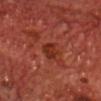Findings:
– imaging modality: ~15 mm tile from a whole-body skin photo
– diameter: ~3 mm (longest diameter)
– tile lighting: cross-polarized
– patient: male, approximately 70 years of age
– TBP lesion metrics: a lesion color around L≈31 a*≈29 b*≈31 in CIELAB, about 8 CIELAB-L* units darker than the surrounding skin, and a normalized border contrast of about 7.5; border irregularity of about 2.5 on a 0–10 scale and peripheral color asymmetry of about 1; a classifier nevus-likeness of about 0/100
– location: the front of the torso